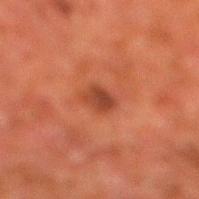Notes:
* workup: total-body-photography surveillance lesion; no biopsy
* subject: male, aged 78 to 82
* acquisition: total-body-photography crop, ~15 mm field of view
* body site: the right lower leg